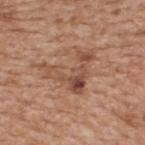| key | value |
|---|---|
| diameter | ~6 mm (longest diameter) |
| imaging modality | ~15 mm crop, total-body skin-cancer survey |
| subject | male, roughly 65 years of age |
| automated metrics | a lesion area of about 12 mm² and a shape eccentricity near 0.7; a border-irregularity rating of about 10/10, a color-variation rating of about 6/10, and radial color variation of about 1.5 |
| tile lighting | white-light |
| location | the upper back |